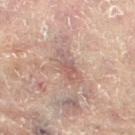This lesion was catalogued during total-body skin photography and was not selected for biopsy. Automated tile analysis of the lesion measured a lesion area of about 8 mm², an eccentricity of roughly 0.9, and a symmetry-axis asymmetry near 0.2. It also reported a lesion color around L≈53 a*≈17 b*≈22 in CIELAB, roughly 8 lightness units darker than nearby skin, and a normalized border contrast of about 5.5. The analysis additionally found border irregularity of about 3 on a 0–10 scale and a peripheral color-asymmetry measure near 2. The software also gave a nevus-likeness score of about 0/100 and lesion-presence confidence of about 55/100. Approximately 4.5 mm at its widest. The lesion is on the left leg. The subject is a female aged 78 to 82. A roughly 15 mm field-of-view crop from a total-body skin photograph.